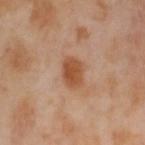notes = imaged on a skin check; not biopsied | imaging modality = ~15 mm crop, total-body skin-cancer survey | lesion size = ≈3.5 mm | patient = female, aged 53–57 | tile lighting = cross-polarized illumination | automated lesion analysis = an automated nevus-likeness rating near 95 out of 100 and lesion-presence confidence of about 100/100 | body site = the left thigh.